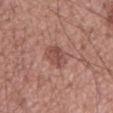Case summary:
* follow-up: total-body-photography surveillance lesion; no biopsy
* subject: male, approximately 55 years of age
* lighting: white-light illumination
* imaging modality: total-body-photography crop, ~15 mm field of view
* body site: the mid back